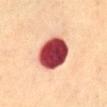No biopsy was performed on this lesion — it was imaged during a full skin examination and was not determined to be concerning. A subject aged 68–72. The tile uses cross-polarized illumination. On the abdomen. Approximately 5 mm at its widest. A 15 mm close-up extracted from a 3D total-body photography capture.